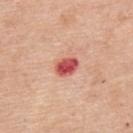Imaged during a routine full-body skin examination; the lesion was not biopsied and no histopathology is available. A lesion tile, about 15 mm wide, cut from a 3D total-body photograph. Automated tile analysis of the lesion measured an area of roughly 5 mm², an outline eccentricity of about 0.7 (0 = round, 1 = elongated), and a shape-asymmetry score of about 0.15 (0 = symmetric). The analysis additionally found a classifier nevus-likeness of about 0/100 and a lesion-detection confidence of about 100/100. A male subject, roughly 55 years of age. From the back. Captured under white-light illumination. About 2.5 mm across.